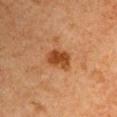On the left upper arm.
The patient is a male aged approximately 65.
Cropped from a whole-body photographic skin survey; the tile spans about 15 mm.
Longest diameter approximately 3 mm.
Automated tile analysis of the lesion measured a lesion area of about 6.5 mm², an outline eccentricity of about 0.6 (0 = round, 1 = elongated), and a shape-asymmetry score of about 0.2 (0 = symmetric). The analysis additionally found an automated nevus-likeness rating near 90 out of 100 and lesion-presence confidence of about 100/100.